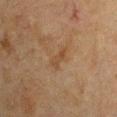Assessment:
Recorded during total-body skin imaging; not selected for excision or biopsy.
Context:
The lesion is on the left upper arm. The tile uses cross-polarized illumination. A male patient, aged 63 to 67. Cropped from a total-body skin-imaging series; the visible field is about 15 mm. Longest diameter approximately 2.5 mm.